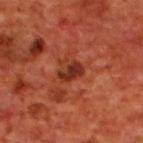The lesion was photographed on a routine skin check and not biopsied; there is no pathology result.